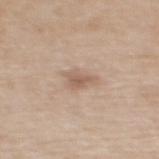workup: total-body-photography surveillance lesion; no biopsy | lesion size: ~3 mm (longest diameter) | patient: female, about 55 years old | site: the upper back | lighting: white-light | automated metrics: an average lesion color of about L≈59 a*≈16 b*≈28 (CIELAB), roughly 9 lightness units darker than nearby skin, and a lesion-to-skin contrast of about 6.5 (normalized; higher = more distinct); a border-irregularity rating of about 4.5/10, internal color variation of about 2 on a 0–10 scale, and peripheral color asymmetry of about 0.5 | image source: ~15 mm tile from a whole-body skin photo.